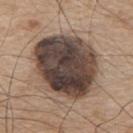Case summary:
– workup · no biopsy performed (imaged during a skin exam)
– subject · male, in their mid-70s
– image · ~15 mm tile from a whole-body skin photo
– lesion diameter · ~8.5 mm (longest diameter)
– automated metrics · a lesion area of about 46 mm², a shape eccentricity near 0.55, and a shape-asymmetry score of about 0.1 (0 = symmetric)
– body site · the upper back
– illumination · white-light